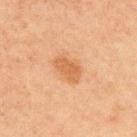Findings:
– subject — male, in their mid- to late 60s
– lesion diameter — ~4 mm (longest diameter)
– TBP lesion metrics — an area of roughly 7.5 mm², an eccentricity of roughly 0.8, and two-axis asymmetry of about 0.2; an average lesion color of about L≈50 a*≈20 b*≈34 (CIELAB) and a normalized border contrast of about 6.5; border irregularity of about 2 on a 0–10 scale, internal color variation of about 2 on a 0–10 scale, and peripheral color asymmetry of about 0.5; an automated nevus-likeness rating near 80 out of 100 and a detector confidence of about 100 out of 100 that the crop contains a lesion
– location — the front of the torso
– acquisition — total-body-photography crop, ~15 mm field of view